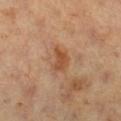Part of a total-body skin-imaging series; this lesion was reviewed on a skin check and was not flagged for biopsy.
Located on the right lower leg.
The lesion's longest dimension is about 3 mm.
A roughly 15 mm field-of-view crop from a total-body skin photograph.
The subject is a female in their 70s.